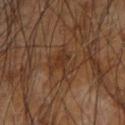Clinical impression:
Part of a total-body skin-imaging series; this lesion was reviewed on a skin check and was not flagged for biopsy.
Context:
Imaged with cross-polarized lighting. The lesion is located on the right forearm. The lesion's longest dimension is about 4 mm. A male patient, aged around 65. A roughly 15 mm field-of-view crop from a total-body skin photograph.